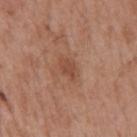{"site": "back", "image": {"source": "total-body photography crop", "field_of_view_mm": 15}, "lesion_size": {"long_diameter_mm_approx": 3.0}, "patient": {"sex": "male", "age_approx": 55}, "lighting": "white-light"}